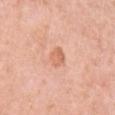Findings:
– workup · no biopsy performed (imaged during a skin exam)
– size · ~2.5 mm (longest diameter)
– lighting · white-light
– location · the left upper arm
– automated metrics · a mean CIELAB color near L≈66 a*≈26 b*≈34, roughly 9 lightness units darker than nearby skin, and a normalized border contrast of about 6; border irregularity of about 2.5 on a 0–10 scale, internal color variation of about 3 on a 0–10 scale, and peripheral color asymmetry of about 1.5; an automated nevus-likeness rating near 15 out of 100 and a lesion-detection confidence of about 100/100
– subject · female, aged 48–52
– image · ~15 mm crop, total-body skin-cancer survey